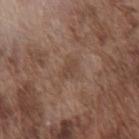Captured during whole-body skin photography for melanoma surveillance; the lesion was not biopsied. From the chest. A lesion tile, about 15 mm wide, cut from a 3D total-body photograph. The patient is a male aged 73–77.